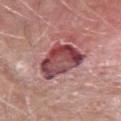Recorded during total-body skin imaging; not selected for excision or biopsy. The lesion is located on the right forearm. A male patient aged approximately 80. Measured at roughly 6.5 mm in maximum diameter. A region of skin cropped from a whole-body photographic capture, roughly 15 mm wide. The tile uses white-light illumination.